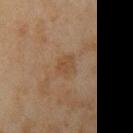Findings:
• notes: total-body-photography surveillance lesion; no biopsy
• imaging modality: ~15 mm crop, total-body skin-cancer survey
• TBP lesion metrics: a border-irregularity index near 2/10 and a peripheral color-asymmetry measure near 1
• illumination: cross-polarized illumination
• location: the right upper arm
• patient: male, aged around 45